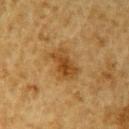{
  "biopsy_status": "not biopsied; imaged during a skin examination",
  "automated_metrics": {
    "area_mm2_approx": 8.5,
    "eccentricity": 0.8,
    "shape_asymmetry": 0.3,
    "vs_skin_darker_L": 9.0,
    "vs_skin_contrast_norm": 7.5,
    "border_irregularity_0_10": 3.5,
    "color_variation_0_10": 4.0
  },
  "lighting": "cross-polarized",
  "patient": {
    "sex": "male",
    "age_approx": "85+"
  },
  "site": "right upper arm",
  "image": {
    "source": "total-body photography crop",
    "field_of_view_mm": 15
  }
}